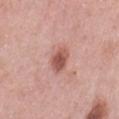<lesion>
  <biopsy_status>not biopsied; imaged during a skin examination</biopsy_status>
  <patient>
    <sex>female</sex>
    <age_approx>40</age_approx>
  </patient>
  <lesion_size>
    <long_diameter_mm_approx>3.0</long_diameter_mm_approx>
  </lesion_size>
  <lighting>white-light</lighting>
  <image>
    <source>total-body photography crop</source>
    <field_of_view_mm>15</field_of_view_mm>
  </image>
  <automated_metrics>
    <area_mm2_approx>6.0</area_mm2_approx>
    <eccentricity>0.7</eccentricity>
    <shape_asymmetry>0.15</shape_asymmetry>
    <cielab_L>55</cielab_L>
    <cielab_a>25</cielab_a>
    <cielab_b>26</cielab_b>
    <vs_skin_darker_L>13.0</vs_skin_darker_L>
    <vs_skin_contrast_norm>8.5</vs_skin_contrast_norm>
    <border_irregularity_0_10>1.5</border_irregularity_0_10>
    <color_variation_0_10>3.5</color_variation_0_10>
  </automated_metrics>
  <site>left thigh</site>
</lesion>